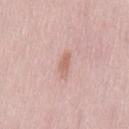The lesion was tiled from a total-body skin photograph and was not biopsied. A female patient, roughly 40 years of age. On the back. A region of skin cropped from a whole-body photographic capture, roughly 15 mm wide. Captured under white-light illumination.